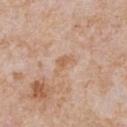- patient — male, aged 63–67
- site — the chest
- diameter — about 3 mm
- imaging modality — ~15 mm tile from a whole-body skin photo
- illumination — white-light